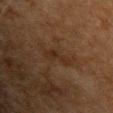Q: Patient demographics?
A: male, in their mid- to late 60s
Q: Where on the body is the lesion?
A: the front of the torso
Q: What kind of image is this?
A: total-body-photography crop, ~15 mm field of view